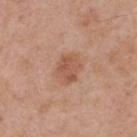  biopsy_status: not biopsied; imaged during a skin examination
  lighting: white-light
  patient:
    sex: male
    age_approx: 55
  automated_metrics:
    area_mm2_approx: 8.0
    eccentricity: 0.65
    shape_asymmetry: 0.2
    cielab_L: 55
    cielab_a: 22
    cielab_b: 31
    vs_skin_darker_L: 9.0
    vs_skin_contrast_norm: 6.5
    border_irregularity_0_10: 2.0
  lesion_size:
    long_diameter_mm_approx: 3.5
  site: upper back
  image:
    source: total-body photography crop
    field_of_view_mm: 15